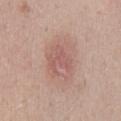Q: Was a biopsy performed?
A: no biopsy performed (imaged during a skin exam)
Q: Automated lesion metrics?
A: a shape-asymmetry score of about 0.5 (0 = symmetric); a border-irregularity index near 7/10 and radial color variation of about 0.5; an automated nevus-likeness rating near 0 out of 100 and lesion-presence confidence of about 100/100
Q: How was the tile lit?
A: white-light
Q: Who is the patient?
A: male, aged around 40
Q: How was this image acquired?
A: ~15 mm crop, total-body skin-cancer survey
Q: What is the anatomic site?
A: the back